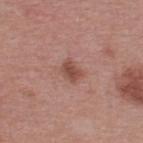biopsy status: imaged on a skin check; not biopsied | automated lesion analysis: an automated nevus-likeness rating near 60 out of 100 and lesion-presence confidence of about 100/100 | subject: male, approximately 40 years of age | imaging modality: ~15 mm tile from a whole-body skin photo | body site: the upper back | lesion size: ≈2.5 mm | lighting: white-light illumination.